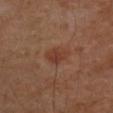Assessment: The lesion was photographed on a routine skin check and not biopsied; there is no pathology result. Background: The lesion is located on the right upper arm. The lesion-visualizer software estimated an outline eccentricity of about 0.7 (0 = round, 1 = elongated). It also reported an average lesion color of about L≈38 a*≈22 b*≈28 (CIELAB) and a lesion–skin lightness drop of about 7. The software also gave a color-variation rating of about 2/10. The analysis additionally found a classifier nevus-likeness of about 10/100. Captured under cross-polarized illumination. This image is a 15 mm lesion crop taken from a total-body photograph. A male subject, approximately 55 years of age. Approximately 3 mm at its widest.